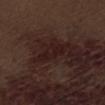Clinical impression:
The lesion was photographed on a routine skin check and not biopsied; there is no pathology result.
Background:
A 15 mm close-up tile from a total-body photography series done for melanoma screening. A male patient, about 70 years old. Located on the abdomen. Approximately 5 mm at its widest.